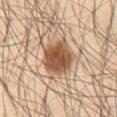Assessment:
The lesion was photographed on a routine skin check and not biopsied; there is no pathology result.
Context:
The subject is a male approximately 65 years of age. This image is a 15 mm lesion crop taken from a total-body photograph. From the abdomen.